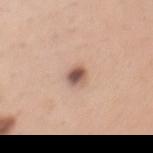Findings:
– follow-up: total-body-photography surveillance lesion; no biopsy
– acquisition: ~15 mm crop, total-body skin-cancer survey
– anatomic site: the chest
– lighting: white-light illumination
– lesion size: ~2.5 mm (longest diameter)
– patient: female, approximately 30 years of age